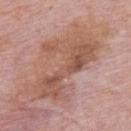site = the upper back
lighting = white-light
diameter = ~9 mm (longest diameter)
subject = male, approximately 75 years of age
acquisition = ~15 mm crop, total-body skin-cancer survey